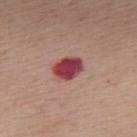follow-up: no biopsy performed (imaged during a skin exam) | subject: male, in their mid- to late 50s | image-analysis metrics: a lesion area of about 8 mm², a shape eccentricity near 0.7, and a symmetry-axis asymmetry near 0.15; a mean CIELAB color near L≈44 a*≈34 b*≈22, roughly 17 lightness units darker than nearby skin, and a normalized border contrast of about 12.5; a classifier nevus-likeness of about 0/100 and a lesion-detection confidence of about 100/100 | image: 15 mm crop, total-body photography | diameter: about 3.5 mm | tile lighting: white-light illumination | site: the upper back.